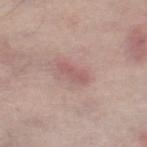  biopsy_status: not biopsied; imaged during a skin examination
  patient:
    sex: male
    age_approx: 65
  lighting: white-light
  automated_metrics:
    area_mm2_approx: 4.5
    eccentricity: 0.9
    shape_asymmetry: 0.3
    peripheral_color_asymmetry: 0.5
    nevus_likeness_0_100: 5
    lesion_detection_confidence_0_100: 100
  site: right lower leg
  lesion_size:
    long_diameter_mm_approx: 3.5
  image:
    source: total-body photography crop
    field_of_view_mm: 15